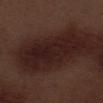Assessment:
Recorded during total-body skin imaging; not selected for excision or biopsy.
Image and clinical context:
The total-body-photography lesion software estimated an area of roughly 26 mm², an eccentricity of roughly 0.9, and a shape-asymmetry score of about 0.25 (0 = symmetric). It also reported a lesion color around L≈19 a*≈18 b*≈17 in CIELAB. A roughly 15 mm field-of-view crop from a total-body skin photograph. The tile uses white-light illumination. The lesion is located on the left thigh. The subject is a male aged 68–72. Measured at roughly 8.5 mm in maximum diameter.